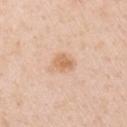Captured during whole-body skin photography for melanoma surveillance; the lesion was not biopsied. The total-body-photography lesion software estimated a lesion–skin lightness drop of about 9 and a lesion-to-skin contrast of about 6.5 (normalized; higher = more distinct). It also reported a color-variation rating of about 2.5/10 and peripheral color asymmetry of about 1. It also reported a nevus-likeness score of about 45/100 and a detector confidence of about 100 out of 100 that the crop contains a lesion. Measured at roughly 2.5 mm in maximum diameter. A male patient approximately 50 years of age. Imaged with white-light lighting. A region of skin cropped from a whole-body photographic capture, roughly 15 mm wide. The lesion is located on the right upper arm.